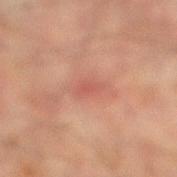Measured at roughly 3 mm in maximum diameter. Captured under cross-polarized illumination. A 15 mm crop from a total-body photograph taken for skin-cancer surveillance. Automated image analysis of the tile measured an area of roughly 3.5 mm². From the leg. A male patient, aged around 75.